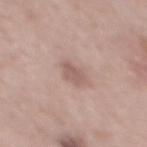<lesion>
  <biopsy_status>not biopsied; imaged during a skin examination</biopsy_status>
  <site>mid back</site>
  <automated_metrics>
    <area_mm2_approx>4.0</area_mm2_approx>
    <eccentricity>0.8</eccentricity>
    <shape_asymmetry>0.25</shape_asymmetry>
    <border_irregularity_0_10>3.0</border_irregularity_0_10>
    <color_variation_0_10>1.5</color_variation_0_10>
    <peripheral_color_asymmetry>0.5</peripheral_color_asymmetry>
    <nevus_likeness_0_100>5</nevus_likeness_0_100>
    <lesion_detection_confidence_0_100>100</lesion_detection_confidence_0_100>
  </automated_metrics>
  <image>
    <source>total-body photography crop</source>
    <field_of_view_mm>15</field_of_view_mm>
  </image>
  <patient>
    <sex>male</sex>
    <age_approx>55</age_approx>
  </patient>
</lesion>